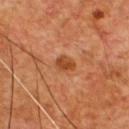Background:
A close-up tile cropped from a whole-body skin photograph, about 15 mm across. Approximately 2.5 mm at its widest. On the chest. A male subject in their mid-50s.